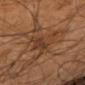Impression: The lesion was tiled from a total-body skin photograph and was not biopsied.